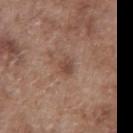The lesion was photographed on a routine skin check and not biopsied; there is no pathology result.
A region of skin cropped from a whole-body photographic capture, roughly 15 mm wide.
The total-body-photography lesion software estimated an area of roughly 4 mm² and a shape-asymmetry score of about 0.3 (0 = symmetric). The analysis additionally found a lesion color around L≈47 a*≈19 b*≈26 in CIELAB, a lesion–skin lightness drop of about 8, and a normalized border contrast of about 6.5. It also reported a nevus-likeness score of about 20/100 and a detector confidence of about 100 out of 100 that the crop contains a lesion.
The recorded lesion diameter is about 2.5 mm.
From the front of the torso.
The patient is a male aged 73 to 77.